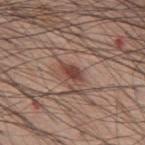Recorded during total-body skin imaging; not selected for excision or biopsy. A region of skin cropped from a whole-body photographic capture, roughly 15 mm wide. Longest diameter approximately 2.5 mm. On the mid back. The lesion-visualizer software estimated an automated nevus-likeness rating near 65 out of 100 and lesion-presence confidence of about 100/100. A male patient aged 58 to 62. The tile uses white-light illumination.